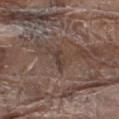| field | value |
|---|---|
| workup | no biopsy performed (imaged during a skin exam) |
| location | the left thigh |
| acquisition | ~15 mm tile from a whole-body skin photo |
| subject | male, aged around 80 |